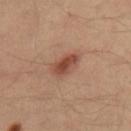Recorded during total-body skin imaging; not selected for excision or biopsy. A roughly 15 mm field-of-view crop from a total-body skin photograph. A male patient aged 38–42. The lesion-visualizer software estimated border irregularity of about 2 on a 0–10 scale and a peripheral color-asymmetry measure near 1. The analysis additionally found lesion-presence confidence of about 100/100. Imaged with cross-polarized lighting. About 3.5 mm across. On the right thigh.